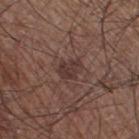| field | value |
|---|---|
| follow-up | total-body-photography surveillance lesion; no biopsy |
| image | ~15 mm tile from a whole-body skin photo |
| subject | male, about 60 years old |
| location | the leg |
| automated lesion analysis | an average lesion color of about L≈35 a*≈18 b*≈20 (CIELAB), about 8 CIELAB-L* units darker than the surrounding skin, and a lesion-to-skin contrast of about 7.5 (normalized; higher = more distinct); lesion-presence confidence of about 100/100 |
| illumination | white-light |
| size | about 3 mm |